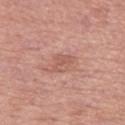Imaged during a routine full-body skin examination; the lesion was not biopsied and no histopathology is available.
Cropped from a whole-body photographic skin survey; the tile spans about 15 mm.
A female subject in their mid-50s.
This is a white-light tile.
The lesion is located on the left thigh.